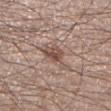The lesion was photographed on a routine skin check and not biopsied; there is no pathology result. An algorithmic analysis of the crop reported an average lesion color of about L≈50 a*≈17 b*≈24 (CIELAB) and roughly 10 lightness units darker than nearby skin. The software also gave a border-irregularity index near 5.5/10, a color-variation rating of about 4/10, and a peripheral color-asymmetry measure near 1.5. The analysis additionally found a classifier nevus-likeness of about 60/100. Cropped from a total-body skin-imaging series; the visible field is about 15 mm. The lesion is on the left lower leg. A male subject, in their 30s. Captured under white-light illumination.